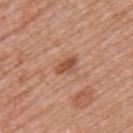notes: imaged on a skin check; not biopsied
image-analysis metrics: a border-irregularity index near 3/10, a color-variation rating of about 3/10, and a peripheral color-asymmetry measure near 1; a nevus-likeness score of about 70/100 and a lesion-detection confidence of about 100/100
imaging modality: 15 mm crop, total-body photography
anatomic site: the upper back
lighting: white-light illumination
subject: female, in their 50s